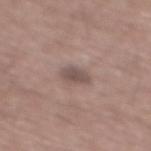Clinical impression:
The lesion was tiled from a total-body skin photograph and was not biopsied.
Acquisition and patient details:
A 15 mm close-up extracted from a 3D total-body photography capture. A male subject, aged 58–62. The lesion's longest dimension is about 2.5 mm. The lesion is located on the mid back.